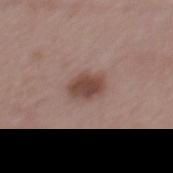Q: Was this lesion biopsied?
A: catalogued during a skin exam; not biopsied
Q: How was this image acquired?
A: ~15 mm tile from a whole-body skin photo
Q: Who is the patient?
A: male, about 55 years old